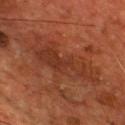Recorded during total-body skin imaging; not selected for excision or biopsy. On the front of the torso. A male patient, roughly 55 years of age. Cropped from a total-body skin-imaging series; the visible field is about 15 mm.